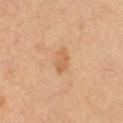Assessment:
The lesion was photographed on a routine skin check and not biopsied; there is no pathology result.
Image and clinical context:
The recorded lesion diameter is about 3.5 mm. A male patient roughly 55 years of age. Cropped from a whole-body photographic skin survey; the tile spans about 15 mm. From the arm.